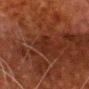Part of a total-body skin-imaging series; this lesion was reviewed on a skin check and was not flagged for biopsy. A roughly 15 mm field-of-view crop from a total-body skin photograph. The patient is a male in their 80s. The lesion is located on the head or neck.